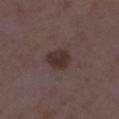  biopsy_status: not biopsied; imaged during a skin examination
  patient:
    sex: female
    age_approx: 35
  image:
    source: total-body photography crop
    field_of_view_mm: 15
  lesion_size:
    long_diameter_mm_approx: 3.5
  site: right lower leg
  lighting: white-light